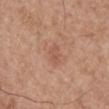* biopsy status · total-body-photography surveillance lesion; no biopsy
* image · 15 mm crop, total-body photography
* lighting · white-light
* subject · male, aged approximately 65
* site · the back
* lesion size · about 2.5 mm